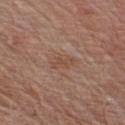biopsy_status: not biopsied; imaged during a skin examination
lighting: white-light
site: front of the torso
image:
  source: total-body photography crop
  field_of_view_mm: 15
patient:
  sex: male
  age_approx: 65
automated_metrics:
  vs_skin_darker_L: 6.0
  vs_skin_contrast_norm: 5.5
  border_irregularity_0_10: 5.0
  color_variation_0_10: 0.0
lesion_size:
  long_diameter_mm_approx: 3.0